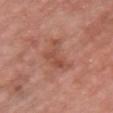Captured under white-light illumination. On the chest. A female patient roughly 40 years of age. Approximately 4.5 mm at its widest. An algorithmic analysis of the crop reported a footprint of about 9 mm², a shape eccentricity near 0.8, and two-axis asymmetry of about 0.55. Cropped from a whole-body photographic skin survey; the tile spans about 15 mm.